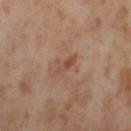workup — no biopsy performed (imaged during a skin exam).